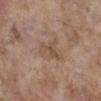Captured during whole-body skin photography for melanoma surveillance; the lesion was not biopsied.
A female subject roughly 85 years of age or older.
This image is a 15 mm lesion crop taken from a total-body photograph.
On the right lower leg.